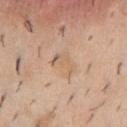Imaged during a routine full-body skin examination; the lesion was not biopsied and no histopathology is available. Measured at roughly 3 mm in maximum diameter. The lesion is on the chest. A close-up tile cropped from a whole-body skin photograph, about 15 mm across. An algorithmic analysis of the crop reported a nevus-likeness score of about 0/100. The subject is a male roughly 40 years of age.